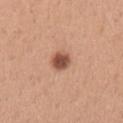Clinical impression: No biopsy was performed on this lesion — it was imaged during a full skin examination and was not determined to be concerning. Clinical summary: Imaged with white-light lighting. A 15 mm close-up extracted from a 3D total-body photography capture. A female patient aged around 30. Located on the arm.